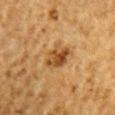Q: Was this lesion biopsied?
A: no biopsy performed (imaged during a skin exam)
Q: What kind of image is this?
A: ~15 mm tile from a whole-body skin photo
Q: Patient demographics?
A: male, in their mid- to late 80s or older
Q: What is the anatomic site?
A: the right upper arm
Q: How large is the lesion?
A: about 3.5 mm